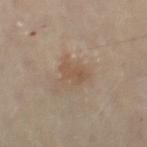{"biopsy_status": "not biopsied; imaged during a skin examination"}